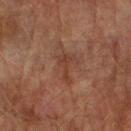biopsy status: imaged on a skin check; not biopsied | site: the right forearm | diameter: ~3.5 mm (longest diameter) | image source: total-body-photography crop, ~15 mm field of view | illumination: cross-polarized | TBP lesion metrics: an area of roughly 4 mm², an outline eccentricity of about 0.85 (0 = round, 1 = elongated), and a shape-asymmetry score of about 0.65 (0 = symmetric); a mean CIELAB color near L≈32 a*≈19 b*≈24 and a normalized border contrast of about 5.5; a border-irregularity index near 7.5/10, a within-lesion color-variation index near 1/10, and radial color variation of about 0.5 | subject: male, aged 73–77.